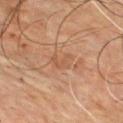Recorded during total-body skin imaging; not selected for excision or biopsy.